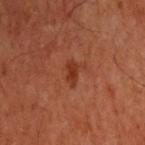<tbp_lesion>
<biopsy_status>not biopsied; imaged during a skin examination</biopsy_status>
<lighting>cross-polarized</lighting>
<patient>
  <sex>male</sex>
  <age_approx>60</age_approx>
</patient>
<image>
  <source>total-body photography crop</source>
  <field_of_view_mm>15</field_of_view_mm>
</image>
<site>head or neck</site>
</tbp_lesion>